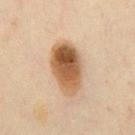Imaged during a routine full-body skin examination; the lesion was not biopsied and no histopathology is available. This image is a 15 mm lesion crop taken from a total-body photograph. A male subject, roughly 45 years of age. On the chest.